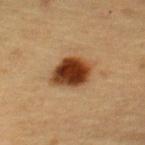This lesion was catalogued during total-body skin photography and was not selected for biopsy. Located on the upper back. This image is a 15 mm lesion crop taken from a total-body photograph. About 4 mm across. A female subject, in their 60s. An algorithmic analysis of the crop reported a lesion color around L≈35 a*≈21 b*≈32 in CIELAB, roughly 18 lightness units darker than nearby skin, and a normalized lesion–skin contrast near 14.